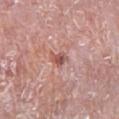Q: Is there a histopathology result?
A: no biopsy performed (imaged during a skin exam)
Q: How was the tile lit?
A: white-light
Q: Patient demographics?
A: male, approximately 75 years of age
Q: Where on the body is the lesion?
A: the leg
Q: What kind of image is this?
A: ~15 mm crop, total-body skin-cancer survey
Q: How large is the lesion?
A: ~2.5 mm (longest diameter)
Q: What did automated image analysis measure?
A: a lesion area of about 3.5 mm², an outline eccentricity of about 0.7 (0 = round, 1 = elongated), and a shape-asymmetry score of about 0.4 (0 = symmetric); a mean CIELAB color near L≈54 a*≈24 b*≈25, about 11 CIELAB-L* units darker than the surrounding skin, and a normalized lesion–skin contrast near 7.5